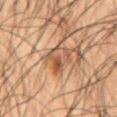biopsy status: imaged on a skin check; not biopsied
illumination: cross-polarized
subject: male, aged around 50
diameter: ≈4 mm
automated metrics: a mean CIELAB color near L≈40 a*≈17 b*≈26, roughly 8 lightness units darker than nearby skin, and a lesion-to-skin contrast of about 7 (normalized; higher = more distinct); a border-irregularity rating of about 3.5/10, internal color variation of about 7.5 on a 0–10 scale, and a peripheral color-asymmetry measure near 2.5
acquisition: 15 mm crop, total-body photography
location: the abdomen